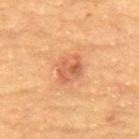lesion_size:
  long_diameter_mm_approx: 3.0
patient:
  sex: female
  age_approx: 70
image:
  source: total-body photography crop
  field_of_view_mm: 15
site: upper back
lighting: cross-polarized